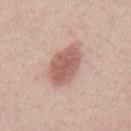Assessment: This lesion was catalogued during total-body skin photography and was not selected for biopsy. Clinical summary: Automated image analysis of the tile measured a nevus-likeness score of about 95/100 and a lesion-detection confidence of about 100/100. The subject is a male approximately 40 years of age. From the mid back. Cropped from a whole-body photographic skin survey; the tile spans about 15 mm. Measured at roughly 5.5 mm in maximum diameter. Imaged with white-light lighting.